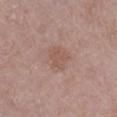Clinical impression:
Part of a total-body skin-imaging series; this lesion was reviewed on a skin check and was not flagged for biopsy.
Image and clinical context:
On the leg. This is a white-light tile. The patient is a male approximately 80 years of age. Measured at roughly 3 mm in maximum diameter. This image is a 15 mm lesion crop taken from a total-body photograph.